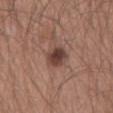Findings:
– notes — total-body-photography surveillance lesion; no biopsy
– lesion diameter — ~3 mm (longest diameter)
– imaging modality — 15 mm crop, total-body photography
– location — the abdomen
– subject — male, approximately 65 years of age
– automated metrics — a footprint of about 6 mm², an eccentricity of roughly 0.5, and two-axis asymmetry of about 0.2; a border-irregularity index near 1.5/10 and radial color variation of about 1.5; a classifier nevus-likeness of about 95/100